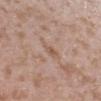Q: Was a biopsy performed?
A: imaged on a skin check; not biopsied
Q: Automated lesion metrics?
A: a lesion area of about 5 mm², an eccentricity of roughly 0.85, and a shape-asymmetry score of about 0.25 (0 = symmetric); an automated nevus-likeness rating near 0 out of 100 and a lesion-detection confidence of about 100/100
Q: Where on the body is the lesion?
A: the right upper arm
Q: How large is the lesion?
A: about 3.5 mm
Q: Illumination type?
A: white-light
Q: What are the patient's age and sex?
A: male, in their mid-40s
Q: What kind of image is this?
A: 15 mm crop, total-body photography